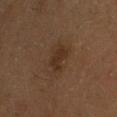| key | value |
|---|---|
| follow-up | catalogued during a skin exam; not biopsied |
| patient | male, in their 60s |
| lesion size | ≈3 mm |
| imaging modality | ~15 mm tile from a whole-body skin photo |
| automated lesion analysis | a classifier nevus-likeness of about 15/100 |
| illumination | cross-polarized illumination |